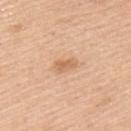anatomic site = the left upper arm
acquisition = ~15 mm crop, total-body skin-cancer survey
lighting = white-light
lesion diameter = about 2.5 mm
patient = male, approximately 50 years of age